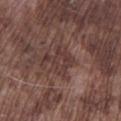Clinical impression:
The lesion was tiled from a total-body skin photograph and was not biopsied.
Context:
A male patient about 75 years old. From the left lower leg. A lesion tile, about 15 mm wide, cut from a 3D total-body photograph.